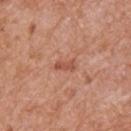{
  "biopsy_status": "not biopsied; imaged during a skin examination",
  "image": {
    "source": "total-body photography crop",
    "field_of_view_mm": 15
  },
  "patient": {
    "sex": "male",
    "age_approx": 65
  },
  "lighting": "white-light",
  "site": "upper back",
  "lesion_size": {
    "long_diameter_mm_approx": 2.5
  }
}